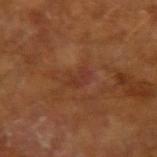No biopsy was performed on this lesion — it was imaged during a full skin examination and was not determined to be concerning.